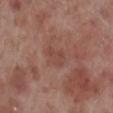Impression: Imaged during a routine full-body skin examination; the lesion was not biopsied and no histopathology is available. Acquisition and patient details: A region of skin cropped from a whole-body photographic capture, roughly 15 mm wide. Captured under white-light illumination. Located on the right lower leg. The subject is a male aged 68–72. About 3 mm across.